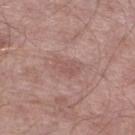follow-up: total-body-photography surveillance lesion; no biopsy
anatomic site: the left lower leg
subject: male, aged 53 to 57
automated lesion analysis: a lesion color around L≈54 a*≈20 b*≈23 in CIELAB, roughly 7 lightness units darker than nearby skin, and a normalized lesion–skin contrast near 4.5; border irregularity of about 4 on a 0–10 scale, internal color variation of about 2 on a 0–10 scale, and a peripheral color-asymmetry measure near 0.5; an automated nevus-likeness rating near 0 out of 100
image source: ~15 mm crop, total-body skin-cancer survey